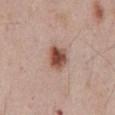A roughly 15 mm field-of-view crop from a total-body skin photograph.
The lesion is located on the abdomen.
A male patient about 55 years old.